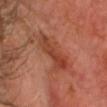Notes:
– follow-up · imaged on a skin check; not biopsied
– lesion diameter · ~5.5 mm (longest diameter)
– automated metrics · an area of roughly 11 mm² and two-axis asymmetry of about 0.4; a border-irregularity rating of about 5/10 and radial color variation of about 2; a nevus-likeness score of about 0/100 and lesion-presence confidence of about 100/100
– image · 15 mm crop, total-body photography
– subject · male, aged approximately 65